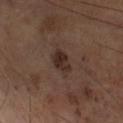Impression:
The lesion was tiled from a total-body skin photograph and was not biopsied.
Clinical summary:
On the right lower leg. A lesion tile, about 15 mm wide, cut from a 3D total-body photograph. The patient is a male about 70 years old.